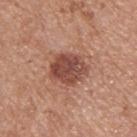Part of a total-body skin-imaging series; this lesion was reviewed on a skin check and was not flagged for biopsy. Located on the upper back. This image is a 15 mm lesion crop taken from a total-body photograph. About 4.5 mm across. A male subject in their mid-50s. The tile uses white-light illumination.